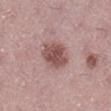{
  "biopsy_status": "not biopsied; imaged during a skin examination"
}